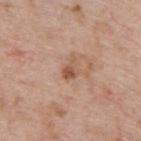The lesion was tiled from a total-body skin photograph and was not biopsied.
A 15 mm close-up tile from a total-body photography series done for melanoma screening.
The recorded lesion diameter is about 2.5 mm.
A male subject, approximately 60 years of age.
The lesion is located on the upper back.
This is a white-light tile.